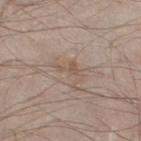The lesion was photographed on a routine skin check and not biopsied; there is no pathology result.
Automated image analysis of the tile measured a lesion color around L≈54 a*≈14 b*≈25 in CIELAB, a lesion–skin lightness drop of about 6, and a lesion-to-skin contrast of about 5 (normalized; higher = more distinct). It also reported a border-irregularity rating of about 7/10. The analysis additionally found a detector confidence of about 100 out of 100 that the crop contains a lesion.
A region of skin cropped from a whole-body photographic capture, roughly 15 mm wide.
From the leg.
The lesion's longest dimension is about 3.5 mm.
Imaged with white-light lighting.
A male subject approximately 60 years of age.